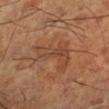Imaged during a routine full-body skin examination; the lesion was not biopsied and no histopathology is available. The tile uses cross-polarized illumination. From the left lower leg. A 15 mm close-up extracted from a 3D total-body photography capture. The patient is a male aged approximately 65.